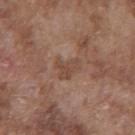patient = male, in their mid-70s; location = the front of the torso; image source = total-body-photography crop, ~15 mm field of view.